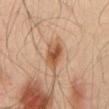The lesion was tiled from a total-body skin photograph and was not biopsied.